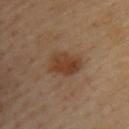notes=no biopsy performed (imaged during a skin exam) | acquisition=15 mm crop, total-body photography | anatomic site=the upper back | lighting=cross-polarized | TBP lesion metrics=a lesion–skin lightness drop of about 9; a classifier nevus-likeness of about 75/100 | subject=male, aged around 40 | size=about 4 mm.